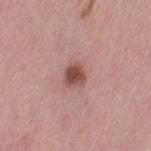No biopsy was performed on this lesion — it was imaged during a full skin examination and was not determined to be concerning.
A female subject, roughly 35 years of age.
Measured at roughly 2.5 mm in maximum diameter.
From the left thigh.
Cropped from a total-body skin-imaging series; the visible field is about 15 mm.
The tile uses white-light illumination.